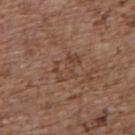Impression:
Imaged during a routine full-body skin examination; the lesion was not biopsied and no histopathology is available.
Acquisition and patient details:
The tile uses white-light illumination. Measured at roughly 3.5 mm in maximum diameter. The subject is a female in their mid- to late 60s. On the upper back. A region of skin cropped from a whole-body photographic capture, roughly 15 mm wide. The total-body-photography lesion software estimated a lesion area of about 6.5 mm² and two-axis asymmetry of about 0.45. And it measured a mean CIELAB color near L≈43 a*≈18 b*≈28. It also reported a border-irregularity index near 6.5/10 and a color-variation rating of about 3.5/10. It also reported a nevus-likeness score of about 0/100.